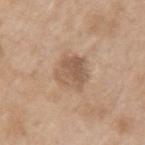• lesion size — about 4 mm
• location — the left upper arm
• lighting — white-light illumination
• TBP lesion metrics — a classifier nevus-likeness of about 5/100
• image — 15 mm crop, total-body photography
• patient — female, aged 73 to 77